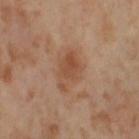– biopsy status: catalogued during a skin exam; not biopsied
– lighting: cross-polarized
– site: the right thigh
– image: ~15 mm crop, total-body skin-cancer survey
– diameter: about 5 mm
– subject: female, aged approximately 55